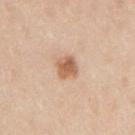lesion_size:
  long_diameter_mm_approx: 2.5
site: arm
patient:
  sex: male
  age_approx: 35
image:
  source: total-body photography crop
  field_of_view_mm: 15
lighting: white-light